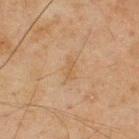Captured during whole-body skin photography for melanoma surveillance; the lesion was not biopsied.
A male patient, aged 43 to 47.
This image is a 15 mm lesion crop taken from a total-body photograph.
Located on the upper back.